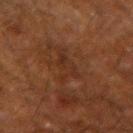{"biopsy_status": "not biopsied; imaged during a skin examination", "image": {"source": "total-body photography crop", "field_of_view_mm": 15}, "site": "left forearm", "lesion_size": {"long_diameter_mm_approx": 4.5}, "patient": {"sex": "male", "age_approx": 60}, "automated_metrics": {"eccentricity": 0.85, "shape_asymmetry": 0.65, "nevus_likeness_0_100": 0, "lesion_detection_confidence_0_100": 85}, "lighting": "cross-polarized"}